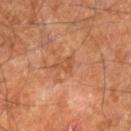Case summary:
- follow-up: total-body-photography surveillance lesion; no biopsy
- size: about 2.5 mm
- TBP lesion metrics: a mean CIELAB color near L≈50 a*≈25 b*≈35, about 7 CIELAB-L* units darker than the surrounding skin, and a lesion-to-skin contrast of about 5 (normalized; higher = more distinct)
- site: the right leg
- patient: male, in their 60s
- illumination: cross-polarized illumination
- image: 15 mm crop, total-body photography